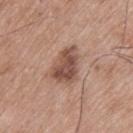No biopsy was performed on this lesion — it was imaged during a full skin examination and was not determined to be concerning.
The lesion is on the upper back.
A male subject aged 73 to 77.
This image is a 15 mm lesion crop taken from a total-body photograph.